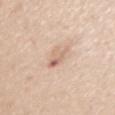This lesion was catalogued during total-body skin photography and was not selected for biopsy. A female subject, in their 40s. Located on the left upper arm. A region of skin cropped from a whole-body photographic capture, roughly 15 mm wide.